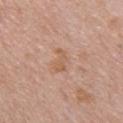notes — catalogued during a skin exam; not biopsied
imaging modality — ~15 mm tile from a whole-body skin photo
location — the upper back
lighting — white-light
TBP lesion metrics — a footprint of about 3 mm², a shape eccentricity near 0.85, and two-axis asymmetry of about 0.55; a lesion–skin lightness drop of about 7 and a normalized border contrast of about 6
subject — male, in their 50s